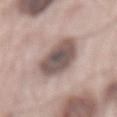notes: total-body-photography surveillance lesion; no biopsy
patient: male, about 65 years old
lesion size: ≈7 mm
image source: 15 mm crop, total-body photography
body site: the mid back